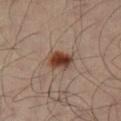Clinical impression: This lesion was catalogued during total-body skin photography and was not selected for biopsy. Acquisition and patient details: Imaged with cross-polarized lighting. A close-up tile cropped from a whole-body skin photograph, about 15 mm across. The subject is a male in their 50s. On the left leg. About 3 mm across.